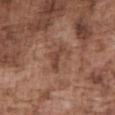Automated tile analysis of the lesion measured an area of roughly 5 mm² and a shape eccentricity near 0.85. The software also gave a nevus-likeness score of about 0/100. From the front of the torso. The recorded lesion diameter is about 3.5 mm. A male subject, aged 73–77. A region of skin cropped from a whole-body photographic capture, roughly 15 mm wide.